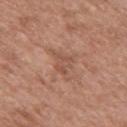Captured during whole-body skin photography for melanoma surveillance; the lesion was not biopsied.
Approximately 2.5 mm at its widest.
The lesion is located on the mid back.
A male patient, approximately 50 years of age.
A 15 mm close-up extracted from a 3D total-body photography capture.